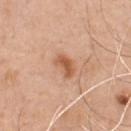Q: Is there a histopathology result?
A: total-body-photography surveillance lesion; no biopsy